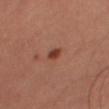This lesion was catalogued during total-body skin photography and was not selected for biopsy.
Cropped from a total-body skin-imaging series; the visible field is about 15 mm.
Measured at roughly 2.5 mm in maximum diameter.
Located on the right thigh.
The patient is a male approximately 60 years of age.
The tile uses cross-polarized illumination.
Automated tile analysis of the lesion measured an area of roughly 2.5 mm², an outline eccentricity of about 0.8 (0 = round, 1 = elongated), and two-axis asymmetry of about 0.2. It also reported about 11 CIELAB-L* units darker than the surrounding skin and a normalized border contrast of about 9.